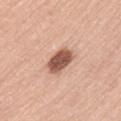About 4 mm across. The tile uses white-light illumination. Cropped from a total-body skin-imaging series; the visible field is about 15 mm. On the lower back. A male patient, in their mid-60s.